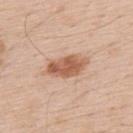biopsy status: total-body-photography surveillance lesion; no biopsy | lesion diameter: ~5 mm (longest diameter) | patient: male, roughly 60 years of age | tile lighting: white-light illumination | image: ~15 mm tile from a whole-body skin photo | anatomic site: the upper back.